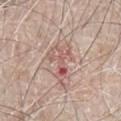Acquisition and patient details:
This is a white-light tile. The lesion is located on the abdomen. Approximately 4.5 mm at its widest. A 15 mm close-up tile from a total-body photography series done for melanoma screening. A male patient aged approximately 65.